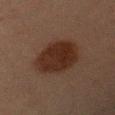biopsy status: no biopsy performed (imaged during a skin exam)
body site: the arm
imaging modality: ~15 mm tile from a whole-body skin photo
lighting: cross-polarized
subject: male, approximately 65 years of age
diameter: about 6.5 mm
TBP lesion metrics: a lesion color around L≈22 a*≈15 b*≈21 in CIELAB and a lesion–skin lightness drop of about 8; a classifier nevus-likeness of about 100/100 and a detector confidence of about 100 out of 100 that the crop contains a lesion